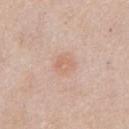{"biopsy_status": "not biopsied; imaged during a skin examination", "image": {"source": "total-body photography crop", "field_of_view_mm": 15}, "lighting": "white-light", "lesion_size": {"long_diameter_mm_approx": 2.5}, "patient": {"sex": "male", "age_approx": 55}, "site": "chest"}